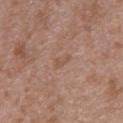Q: How was this image acquired?
A: ~15 mm tile from a whole-body skin photo
Q: Illumination type?
A: white-light
Q: Patient demographics?
A: female, in their 30s
Q: What is the lesion's diameter?
A: ≈2.5 mm
Q: Lesion location?
A: the upper back
Q: Automated lesion metrics?
A: a mean CIELAB color near L≈53 a*≈19 b*≈28, about 6 CIELAB-L* units darker than the surrounding skin, and a normalized lesion–skin contrast near 5